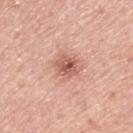follow-up: total-body-photography surveillance lesion; no biopsy | size: about 3 mm | site: the upper back | image source: ~15 mm crop, total-body skin-cancer survey | automated metrics: a symmetry-axis asymmetry near 0.2; an automated nevus-likeness rating near 50 out of 100 and lesion-presence confidence of about 100/100 | illumination: white-light | subject: male, in their mid- to late 60s.